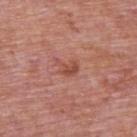The lesion was photographed on a routine skin check and not biopsied; there is no pathology result. A 15 mm close-up tile from a total-body photography series done for melanoma screening. The patient is a male in their mid-70s. From the upper back.